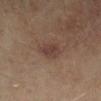No biopsy was performed on this lesion — it was imaged during a full skin examination and was not determined to be concerning. On the leg. An algorithmic analysis of the crop reported an area of roughly 6 mm², an eccentricity of roughly 0.8, and a shape-asymmetry score of about 0.25 (0 = symmetric). Measured at roughly 3.5 mm in maximum diameter. Captured under cross-polarized illumination. A region of skin cropped from a whole-body photographic capture, roughly 15 mm wide. The patient is roughly 60 years of age.